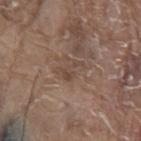follow-up = imaged on a skin check; not biopsied | lesion size = ≈3 mm | patient = male, aged around 80 | location = the front of the torso | lighting = white-light | image source = 15 mm crop, total-body photography.